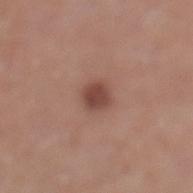follow-up=no biopsy performed (imaged during a skin exam)